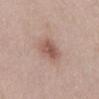This lesion was catalogued during total-body skin photography and was not selected for biopsy. Approximately 3 mm at its widest. Automated tile analysis of the lesion measured a lesion–skin lightness drop of about 11 and a normalized lesion–skin contrast near 7.5. It also reported a classifier nevus-likeness of about 80/100 and lesion-presence confidence of about 100/100. Imaged with white-light lighting. The lesion is located on the abdomen. A female subject aged around 30. Cropped from a whole-body photographic skin survey; the tile spans about 15 mm.